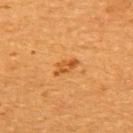Part of a total-body skin-imaging series; this lesion was reviewed on a skin check and was not flagged for biopsy.
Cropped from a total-body skin-imaging series; the visible field is about 15 mm.
A female patient, aged approximately 55.
The lesion is located on the upper back.